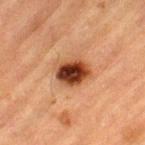Notes:
– biopsy status · total-body-photography surveillance lesion; no biopsy
– body site · the left thigh
– acquisition · 15 mm crop, total-body photography
– tile lighting · cross-polarized illumination
– patient · male, aged approximately 85 or older